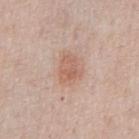notes = imaged on a skin check; not biopsied
subject = male, about 55 years old
illumination = white-light illumination
diameter = about 3.5 mm
image source = ~15 mm crop, total-body skin-cancer survey
automated lesion analysis = roughly 8 lightness units darker than nearby skin and a normalized lesion–skin contrast near 6; a border-irregularity rating of about 2.5/10 and internal color variation of about 3 on a 0–10 scale; a nevus-likeness score of about 80/100 and lesion-presence confidence of about 100/100
location = the front of the torso